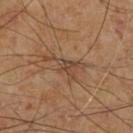Assessment:
Recorded during total-body skin imaging; not selected for excision or biopsy.
Background:
The tile uses cross-polarized illumination. Automated tile analysis of the lesion measured a footprint of about 6.5 mm², an outline eccentricity of about 0.9 (0 = round, 1 = elongated), and two-axis asymmetry of about 0.65. And it measured a lesion color around L≈43 a*≈17 b*≈29 in CIELAB, roughly 7 lightness units darker than nearby skin, and a normalized lesion–skin contrast near 6.5. The software also gave a border-irregularity rating of about 9/10, a within-lesion color-variation index near 3/10, and a peripheral color-asymmetry measure near 1. Cropped from a whole-body photographic skin survey; the tile spans about 15 mm. On the right lower leg. A male subject, aged around 70.